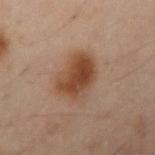workup: total-body-photography surveillance lesion; no biopsy | site: the right upper arm | tile lighting: cross-polarized | automated metrics: border irregularity of about 2.5 on a 0–10 scale, a within-lesion color-variation index near 4/10, and peripheral color asymmetry of about 1.5 | lesion size: ≈4.5 mm | patient: male, roughly 50 years of age | image source: total-body-photography crop, ~15 mm field of view.